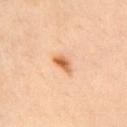image = ~15 mm tile from a whole-body skin photo | patient = male, in their 50s | anatomic site = the chest.